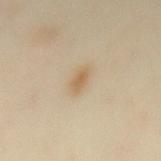Impression: The lesion was photographed on a routine skin check and not biopsied; there is no pathology result. Context: The lesion is located on the mid back. Captured under cross-polarized illumination. A lesion tile, about 15 mm wide, cut from a 3D total-body photograph. A female patient aged 38 to 42. Approximately 3.5 mm at its widest. The total-body-photography lesion software estimated a shape eccentricity near 0.9 and a symmetry-axis asymmetry near 0.2.